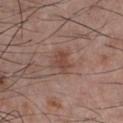Case summary:
- lesion size — about 3 mm
- TBP lesion metrics — an automated nevus-likeness rating near 15 out of 100
- subject — male, aged around 40
- acquisition — ~15 mm tile from a whole-body skin photo
- body site — the chest
- illumination — white-light illumination